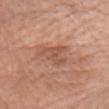Impression: The lesion was tiled from a total-body skin photograph and was not biopsied. Background: On the head or neck. A close-up tile cropped from a whole-body skin photograph, about 15 mm across. The total-body-photography lesion software estimated an area of roughly 7 mm², a shape eccentricity near 0.8, and a symmetry-axis asymmetry near 0.6. The analysis additionally found a lesion color around L≈54 a*≈23 b*≈31 in CIELAB, roughly 8 lightness units darker than nearby skin, and a lesion-to-skin contrast of about 5.5 (normalized; higher = more distinct). The software also gave a nevus-likeness score of about 5/100 and lesion-presence confidence of about 100/100. The patient is a female aged 63–67. Captured under white-light illumination.